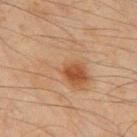No biopsy was performed on this lesion — it was imaged during a full skin examination and was not determined to be concerning. A male subject, aged approximately 45. A 15 mm close-up tile from a total-body photography series done for melanoma screening. The tile uses cross-polarized illumination. The lesion is on the back. Automated image analysis of the tile measured a lesion area of about 24 mm², an eccentricity of roughly 0.35, and a symmetry-axis asymmetry near 0.7. The analysis additionally found a normalized lesion–skin contrast near 5. And it measured a nevus-likeness score of about 75/100 and a detector confidence of about 100 out of 100 that the crop contains a lesion. About 7 mm across.